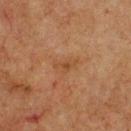<tbp_lesion>
  <biopsy_status>not biopsied; imaged during a skin examination</biopsy_status>
  <image>
    <source>total-body photography crop</source>
    <field_of_view_mm>15</field_of_view_mm>
  </image>
  <site>chest</site>
  <patient>
    <sex>male</sex>
    <age_approx>75</age_approx>
  </patient>
  <lighting>cross-polarized</lighting>
</tbp_lesion>